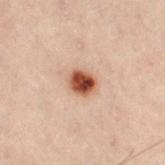Notes:
- biopsy status · catalogued during a skin exam; not biopsied
- automated metrics · a footprint of about 6 mm², a shape eccentricity near 0.55, and a shape-asymmetry score of about 0.1 (0 = symmetric); a border-irregularity index near 1/10, a within-lesion color-variation index near 6.5/10, and peripheral color asymmetry of about 2; a classifier nevus-likeness of about 100/100 and a lesion-detection confidence of about 100/100
- acquisition · ~15 mm crop, total-body skin-cancer survey
- site · the left leg
- subject · male, approximately 50 years of age
- lighting · cross-polarized illumination
- diameter · ~3 mm (longest diameter)